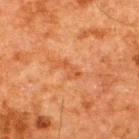Q: Is there a histopathology result?
A: total-body-photography surveillance lesion; no biopsy
Q: Patient demographics?
A: male, aged 58–62
Q: Lesion location?
A: the upper back
Q: How was this image acquired?
A: ~15 mm tile from a whole-body skin photo